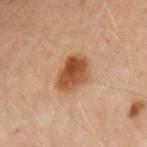This lesion was catalogued during total-body skin photography and was not selected for biopsy. A 15 mm close-up tile from a total-body photography series done for melanoma screening. Approximately 4 mm at its widest. A female patient, aged 28–32. An algorithmic analysis of the crop reported a lesion area of about 11 mm², an eccentricity of roughly 0.4, and two-axis asymmetry of about 0.2. And it measured an average lesion color of about L≈40 a*≈19 b*≈30 (CIELAB), a lesion–skin lightness drop of about 11, and a normalized lesion–skin contrast near 10. It also reported an automated nevus-likeness rating near 100 out of 100. On the left arm.